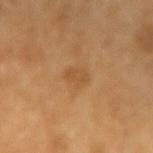Findings:
– biopsy status · total-body-photography surveillance lesion; no biopsy
– anatomic site · the left forearm
– image-analysis metrics · a mean CIELAB color near L≈53 a*≈22 b*≈40 and a normalized border contrast of about 5
– lighting · cross-polarized
– image · ~15 mm crop, total-body skin-cancer survey
– patient · female, roughly 70 years of age
– lesion diameter · ≈3 mm